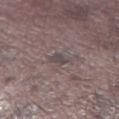Clinical impression:
Recorded during total-body skin imaging; not selected for excision or biopsy.
Background:
A 15 mm close-up tile from a total-body photography series done for melanoma screening. A male patient, aged approximately 75. The recorded lesion diameter is about 2.5 mm. On the leg.